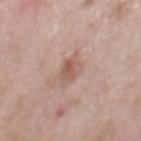{
  "biopsy_status": "not biopsied; imaged during a skin examination",
  "lighting": "white-light",
  "automated_metrics": {
    "cielab_L": 57,
    "cielab_a": 19,
    "cielab_b": 26,
    "vs_skin_contrast_norm": 6.5,
    "border_irregularity_0_10": 2.5,
    "color_variation_0_10": 4.5,
    "peripheral_color_asymmetry": 1.5
  },
  "lesion_size": {
    "long_diameter_mm_approx": 3.5
  },
  "site": "chest",
  "image": {
    "source": "total-body photography crop",
    "field_of_view_mm": 15
  },
  "patient": {
    "sex": "male",
    "age_approx": 50
  }
}